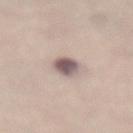Clinical impression:
Recorded during total-body skin imaging; not selected for excision or biopsy.
Context:
Captured under white-light illumination. The lesion's longest dimension is about 3 mm. The patient is a female aged approximately 65. The lesion is located on the abdomen. A 15 mm crop from a total-body photograph taken for skin-cancer surveillance. An algorithmic analysis of the crop reported a lesion area of about 6 mm² and a shape-asymmetry score of about 0.2 (0 = symmetric). It also reported a mean CIELAB color near L≈56 a*≈15 b*≈16, roughly 16 lightness units darker than nearby skin, and a normalized lesion–skin contrast near 11. The software also gave a lesion-detection confidence of about 100/100.